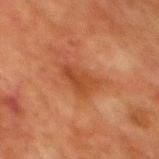Findings:
* acquisition · total-body-photography crop, ~15 mm field of view
* image-analysis metrics · a footprint of about 6.5 mm², an outline eccentricity of about 0.8 (0 = round, 1 = elongated), and a shape-asymmetry score of about 0.35 (0 = symmetric); a lesion color around L≈36 a*≈24 b*≈32 in CIELAB and roughly 7 lightness units darker than nearby skin; a detector confidence of about 100 out of 100 that the crop contains a lesion
* illumination · cross-polarized illumination
* site · the mid back
* patient · male, about 80 years old